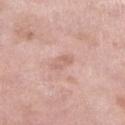No biopsy was performed on this lesion — it was imaged during a full skin examination and was not determined to be concerning.
From the leg.
The tile uses white-light illumination.
The subject is a female aged around 70.
The recorded lesion diameter is about 3 mm.
An algorithmic analysis of the crop reported a lesion color around L≈63 a*≈21 b*≈26 in CIELAB, about 8 CIELAB-L* units darker than the surrounding skin, and a normalized lesion–skin contrast near 5. The analysis additionally found an automated nevus-likeness rating near 0 out of 100 and a detector confidence of about 100 out of 100 that the crop contains a lesion.
Cropped from a whole-body photographic skin survey; the tile spans about 15 mm.